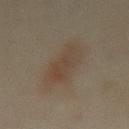biopsy_status: not biopsied; imaged during a skin examination
image:
  source: total-body photography crop
  field_of_view_mm: 15
automated_metrics:
  cielab_L: 40
  cielab_a: 12
  cielab_b: 24
  vs_skin_darker_L: 6.0
  vs_skin_contrast_norm: 6.0
  border_irregularity_0_10: 3.5
  peripheral_color_asymmetry: 1.5
lesion_size:
  long_diameter_mm_approx: 7.0
site: mid back
lighting: cross-polarized
patient:
  sex: female
  age_approx: 35